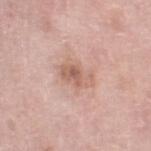Assessment: The lesion was tiled from a total-body skin photograph and was not biopsied. Background: The lesion-visualizer software estimated a footprint of about 9 mm² and an eccentricity of roughly 0.7. The analysis additionally found about 9 CIELAB-L* units darker than the surrounding skin. And it measured a nevus-likeness score of about 15/100 and a detector confidence of about 100 out of 100 that the crop contains a lesion. From the right thigh. Imaged with white-light lighting. A female subject, aged around 60. A lesion tile, about 15 mm wide, cut from a 3D total-body photograph.